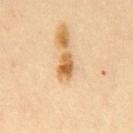Case summary:
- workup · no biopsy performed (imaged during a skin exam)
- anatomic site · the chest
- tile lighting · cross-polarized
- patient · male, in their mid-30s
- automated lesion analysis · a lesion area of about 4.5 mm², a shape eccentricity near 0.8, and a shape-asymmetry score of about 0.3 (0 = symmetric); a mean CIELAB color near L≈56 a*≈19 b*≈39, about 13 CIELAB-L* units darker than the surrounding skin, and a normalized lesion–skin contrast near 10; a classifier nevus-likeness of about 75/100 and a lesion-detection confidence of about 100/100
- imaging modality · total-body-photography crop, ~15 mm field of view
- size · about 3 mm